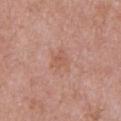Acquisition and patient details: The lesion is located on the chest. A male subject aged 48 to 52. A 15 mm close-up extracted from a 3D total-body photography capture.